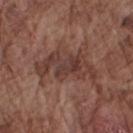follow-up = no biopsy performed (imaged during a skin exam) | body site = the arm | patient = male, about 75 years old | tile lighting = white-light illumination | imaging modality = total-body-photography crop, ~15 mm field of view.